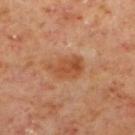Clinical impression:
Imaged during a routine full-body skin examination; the lesion was not biopsied and no histopathology is available.
Image and clinical context:
A 15 mm close-up extracted from a 3D total-body photography capture. A male subject aged around 60. Located on the left lower leg.